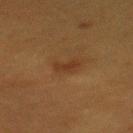Notes:
- workup — imaged on a skin check; not biopsied